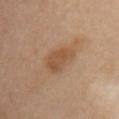Recorded during total-body skin imaging; not selected for excision or biopsy.
Imaged with cross-polarized lighting.
A 15 mm crop from a total-body photograph taken for skin-cancer surveillance.
From the front of the torso.
A female patient, approximately 40 years of age.
Approximately 4 mm at its widest.